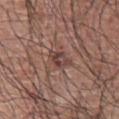Background: The subject is a male aged 68 to 72. Captured under white-light illumination. From the left arm. A 15 mm close-up tile from a total-body photography series done for melanoma screening. The lesion-visualizer software estimated an area of roughly 5.5 mm² and a shape-asymmetry score of about 0.3 (0 = symmetric). The analysis additionally found a border-irregularity rating of about 3/10 and a peripheral color-asymmetry measure near 2. Approximately 3.5 mm at its widest.